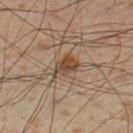Q: Was this lesion biopsied?
A: imaged on a skin check; not biopsied
Q: Lesion size?
A: about 3.5 mm
Q: Automated lesion metrics?
A: an area of roughly 5 mm², a shape eccentricity near 0.75, and a symmetry-axis asymmetry near 0.4
Q: Lesion location?
A: the left lower leg
Q: Illumination type?
A: cross-polarized illumination
Q: What are the patient's age and sex?
A: male, in their mid-50s
Q: What is the imaging modality?
A: ~15 mm crop, total-body skin-cancer survey